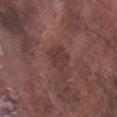Part of a total-body skin-imaging series; this lesion was reviewed on a skin check and was not flagged for biopsy. A male subject aged approximately 75. The lesion's longest dimension is about 3 mm. The tile uses white-light illumination. Located on the right lower leg. The total-body-photography lesion software estimated a footprint of about 6 mm² and an eccentricity of roughly 0.5. The software also gave border irregularity of about 3 on a 0–10 scale, a within-lesion color-variation index near 2.5/10, and a peripheral color-asymmetry measure near 1. Cropped from a whole-body photographic skin survey; the tile spans about 15 mm.